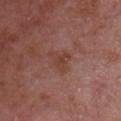Findings:
• follow-up · imaged on a skin check; not biopsied
• lighting · white-light illumination
• image-analysis metrics · a mean CIELAB color near L≈41 a*≈23 b*≈26 and a lesion-to-skin contrast of about 6 (normalized; higher = more distinct); a border-irregularity index near 5/10, internal color variation of about 2 on a 0–10 scale, and radial color variation of about 0.5; a detector confidence of about 100 out of 100 that the crop contains a lesion
• lesion diameter · ~3.5 mm (longest diameter)
• anatomic site · the chest
• patient · male, aged 63 to 67
• imaging modality · 15 mm crop, total-body photography